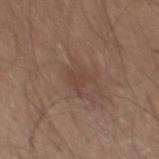<record>
  <biopsy_status>not biopsied; imaged during a skin examination</biopsy_status>
  <patient>
    <sex>male</sex>
    <age_approx>25</age_approx>
  </patient>
  <lighting>white-light</lighting>
  <site>mid back</site>
  <image>
    <source>total-body photography crop</source>
    <field_of_view_mm>15</field_of_view_mm>
  </image>
</record>